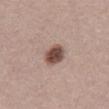Recorded during total-body skin imaging; not selected for excision or biopsy. Approximately 3.5 mm at its widest. A 15 mm crop from a total-body photograph taken for skin-cancer surveillance. The tile uses white-light illumination. A male patient aged 28 to 32.